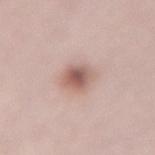Q: Was this lesion biopsied?
A: total-body-photography surveillance lesion; no biopsy
Q: Where on the body is the lesion?
A: the lower back
Q: What kind of image is this?
A: ~15 mm tile from a whole-body skin photo
Q: Patient demographics?
A: female, aged around 40